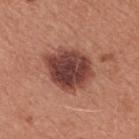Imaged during a routine full-body skin examination; the lesion was not biopsied and no histopathology is available.
The recorded lesion diameter is about 6 mm.
From the front of the torso.
A close-up tile cropped from a whole-body skin photograph, about 15 mm across.
The patient is a female aged approximately 35.